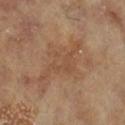patient:
  sex: female
  age_approx: 75
lesion_size:
  long_diameter_mm_approx: 6.5
automated_metrics:
  area_mm2_approx: 10.0
  eccentricity: 0.9
  cielab_L: 48
  cielab_a: 19
  cielab_b: 32
  vs_skin_darker_L: 6.0
  vs_skin_contrast_norm: 4.5
  border_irregularity_0_10: 9.0
  color_variation_0_10: 2.5
  peripheral_color_asymmetry: 0.5
lighting: cross-polarized
site: left lower leg
image:
  source: total-body photography crop
  field_of_view_mm: 15